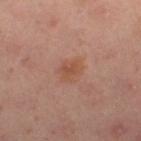Q: Was a biopsy performed?
A: total-body-photography surveillance lesion; no biopsy
Q: Lesion size?
A: ≈3 mm
Q: What is the anatomic site?
A: the leg
Q: What did automated image analysis measure?
A: a footprint of about 5 mm², a shape eccentricity near 0.55, and a symmetry-axis asymmetry near 0.25; a nevus-likeness score of about 30/100 and a lesion-detection confidence of about 100/100
Q: How was the tile lit?
A: cross-polarized
Q: What are the patient's age and sex?
A: female, aged 38 to 42
Q: What is the imaging modality?
A: total-body-photography crop, ~15 mm field of view